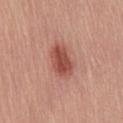subject — male, approximately 55 years of age
image-analysis metrics — a color-variation rating of about 4/10 and peripheral color asymmetry of about 1.5; an automated nevus-likeness rating near 100 out of 100
site — the lower back
image source — ~15 mm tile from a whole-body skin photo